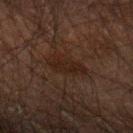  biopsy_status: not biopsied; imaged during a skin examination
  patient:
    sex: male
    age_approx: 50
  image:
    source: total-body photography crop
    field_of_view_mm: 15
  site: left forearm
  lesion_size:
    long_diameter_mm_approx: 4.5